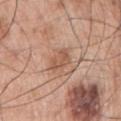Clinical summary: Cropped from a total-body skin-imaging series; the visible field is about 15 mm. Automated image analysis of the tile measured a mean CIELAB color near L≈55 a*≈21 b*≈30 and about 9 CIELAB-L* units darker than the surrounding skin. It also reported a border-irregularity index near 2.5/10, a color-variation rating of about 2.5/10, and a peripheral color-asymmetry measure near 1. Longest diameter approximately 3 mm. A male patient, roughly 70 years of age. On the left arm.